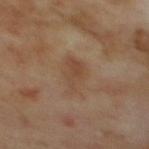Clinical impression: No biopsy was performed on this lesion — it was imaged during a full skin examination and was not determined to be concerning. Context: A lesion tile, about 15 mm wide, cut from a 3D total-body photograph. An algorithmic analysis of the crop reported an average lesion color of about L≈44 a*≈16 b*≈29 (CIELAB) and a normalized lesion–skin contrast near 5. The analysis additionally found a border-irregularity rating of about 3.5/10 and peripheral color asymmetry of about 1. The recorded lesion diameter is about 4.5 mm. The lesion is on the mid back. A male subject, aged around 70. This is a cross-polarized tile.